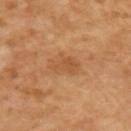Q: Who is the patient?
A: male, approximately 65 years of age
Q: How was the tile lit?
A: cross-polarized
Q: What did automated image analysis measure?
A: a border-irregularity rating of about 4/10, a within-lesion color-variation index near 2/10, and peripheral color asymmetry of about 1
Q: What kind of image is this?
A: total-body-photography crop, ~15 mm field of view
Q: What is the lesion's diameter?
A: ~4 mm (longest diameter)